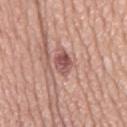Clinical impression:
Recorded during total-body skin imaging; not selected for excision or biopsy.
Background:
An algorithmic analysis of the crop reported a lesion area of about 5 mm², a shape eccentricity near 0.75, and a symmetry-axis asymmetry near 0.2. It also reported a mean CIELAB color near L≈53 a*≈24 b*≈23, about 12 CIELAB-L* units darker than the surrounding skin, and a normalized border contrast of about 8.5. The analysis additionally found a detector confidence of about 100 out of 100 that the crop contains a lesion. A male subject about 75 years old. About 3.5 mm across. On the mid back. Captured under white-light illumination. Cropped from a total-body skin-imaging series; the visible field is about 15 mm.